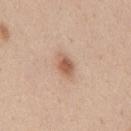This image is a 15 mm lesion crop taken from a total-body photograph. The lesion's longest dimension is about 3 mm. A female patient aged around 40. From the mid back. Captured under white-light illumination.